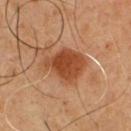Clinical impression: The lesion was photographed on a routine skin check and not biopsied; there is no pathology result. Acquisition and patient details: Cropped from a total-body skin-imaging series; the visible field is about 15 mm. The lesion is on the chest. Imaged with cross-polarized lighting. A male patient, aged 48 to 52.